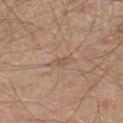The lesion is located on the left lower leg. The patient is a male aged 58 to 62. Cropped from a whole-body photographic skin survey; the tile spans about 15 mm.